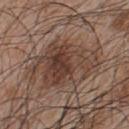No biopsy was performed on this lesion — it was imaged during a full skin examination and was not determined to be concerning. Automated image analysis of the tile measured a lesion area of about 23 mm² and two-axis asymmetry of about 0.5. And it measured an average lesion color of about L≈40 a*≈17 b*≈24 (CIELAB), about 9 CIELAB-L* units darker than the surrounding skin, and a lesion-to-skin contrast of about 8 (normalized; higher = more distinct). It also reported a border-irregularity index near 7.5/10 and peripheral color asymmetry of about 2. And it measured a nevus-likeness score of about 75/100 and lesion-presence confidence of about 100/100. Located on the upper back. Imaged with white-light lighting. Cropped from a whole-body photographic skin survey; the tile spans about 15 mm. A male patient aged 43 to 47. About 7.5 mm across.